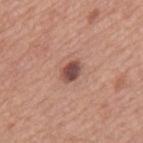Part of a total-body skin-imaging series; this lesion was reviewed on a skin check and was not flagged for biopsy.
From the mid back.
A male patient, aged approximately 75.
A 15 mm close-up tile from a total-body photography series done for melanoma screening.
Captured under white-light illumination.